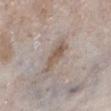No biopsy was performed on this lesion — it was imaged during a full skin examination and was not determined to be concerning. Cropped from a whole-body photographic skin survey; the tile spans about 15 mm. This is a white-light tile. On the left lower leg. A male patient aged 78 to 82. Longest diameter approximately 4.5 mm.